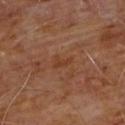Q: Is there a histopathology result?
A: imaged on a skin check; not biopsied
Q: Lesion location?
A: the chest
Q: Lesion size?
A: ~2.5 mm (longest diameter)
Q: What kind of image is this?
A: ~15 mm crop, total-body skin-cancer survey
Q: Automated lesion metrics?
A: a nevus-likeness score of about 0/100 and a detector confidence of about 100 out of 100 that the crop contains a lesion
Q: Who is the patient?
A: male, aged 58–62
Q: How was the tile lit?
A: cross-polarized